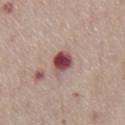The lesion was tiled from a total-body skin photograph and was not biopsied.
Cropped from a total-body skin-imaging series; the visible field is about 15 mm.
The tile uses white-light illumination.
On the chest.
The subject is a male approximately 75 years of age.
The lesion's longest dimension is about 3 mm.
Automated tile analysis of the lesion measured a lesion area of about 6 mm². The software also gave a border-irregularity index near 1.5/10, internal color variation of about 8 on a 0–10 scale, and a peripheral color-asymmetry measure near 2.5.